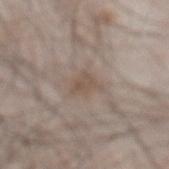<case>
<biopsy_status>not biopsied; imaged during a skin examination</biopsy_status>
<lighting>white-light</lighting>
<site>abdomen</site>
<image>
  <source>total-body photography crop</source>
  <field_of_view_mm>15</field_of_view_mm>
</image>
<patient>
  <sex>male</sex>
  <age_approx>55</age_approx>
</patient>
</case>